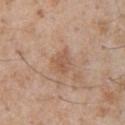Findings:
• biopsy status — no biopsy performed (imaged during a skin exam)
• image — ~15 mm crop, total-body skin-cancer survey
• automated metrics — an eccentricity of roughly 0.65 and two-axis asymmetry of about 0.35; a lesion color around L≈55 a*≈19 b*≈31 in CIELAB, a lesion–skin lightness drop of about 8, and a normalized lesion–skin contrast near 5.5; a border-irregularity rating of about 3/10, a within-lesion color-variation index near 2.5/10, and peripheral color asymmetry of about 1; an automated nevus-likeness rating near 0 out of 100 and a lesion-detection confidence of about 100/100
• patient — male, aged 58–62
• body site — the front of the torso